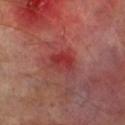This lesion was catalogued during total-body skin photography and was not selected for biopsy. An algorithmic analysis of the crop reported a lesion area of about 7 mm², an eccentricity of roughly 0.65, and a shape-asymmetry score of about 0.25 (0 = symmetric). The software also gave an automated nevus-likeness rating near 0 out of 100 and a detector confidence of about 100 out of 100 that the crop contains a lesion. Longest diameter approximately 3.5 mm. On the leg. A male patient approximately 70 years of age. This image is a 15 mm lesion crop taken from a total-body photograph. Captured under cross-polarized illumination.